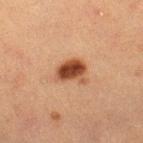Notes:
• workup · imaged on a skin check; not biopsied
• site · the right lower leg
• imaging modality · total-body-photography crop, ~15 mm field of view
• subject · female, aged 53–57
• tile lighting · cross-polarized